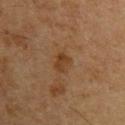Part of a total-body skin-imaging series; this lesion was reviewed on a skin check and was not flagged for biopsy. The patient is a male about 60 years old. A lesion tile, about 15 mm wide, cut from a 3D total-body photograph. Approximately 3 mm at its widest. Imaged with cross-polarized lighting.